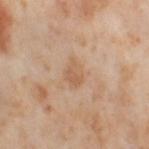<record>
<biopsy_status>not biopsied; imaged during a skin examination</biopsy_status>
<patient>
  <sex>female</sex>
  <age_approx>55</age_approx>
</patient>
<site>right thigh</site>
<lesion_size>
  <long_diameter_mm_approx>3.5</long_diameter_mm_approx>
</lesion_size>
<lighting>cross-polarized</lighting>
<image>
  <source>total-body photography crop</source>
  <field_of_view_mm>15</field_of_view_mm>
</image>
</record>